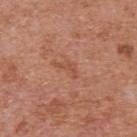Captured during whole-body skin photography for melanoma surveillance; the lesion was not biopsied.